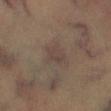Clinical impression:
No biopsy was performed on this lesion — it was imaged during a full skin examination and was not determined to be concerning.
Image and clinical context:
An algorithmic analysis of the crop reported a lesion area of about 4.5 mm² and a shape eccentricity near 0.7. The software also gave an average lesion color of about L≈38 a*≈12 b*≈19 (CIELAB), about 5 CIELAB-L* units darker than the surrounding skin, and a normalized border contrast of about 5. The analysis additionally found a border-irregularity index near 2.5/10, a within-lesion color-variation index near 1.5/10, and peripheral color asymmetry of about 0.5. The analysis additionally found an automated nevus-likeness rating near 5 out of 100 and lesion-presence confidence of about 100/100. A male patient, approximately 45 years of age. A region of skin cropped from a whole-body photographic capture, roughly 15 mm wide. Longest diameter approximately 3 mm. The lesion is on the right lower leg.